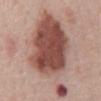No biopsy was performed on this lesion — it was imaged during a full skin examination and was not determined to be concerning. The patient is a male approximately 70 years of age. Measured at roughly 12 mm in maximum diameter. Captured under white-light illumination. A close-up tile cropped from a whole-body skin photograph, about 15 mm across. On the chest.